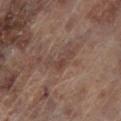This lesion was catalogued during total-body skin photography and was not selected for biopsy. From the left lower leg. A male patient, aged 63–67. A 15 mm crop from a total-body photograph taken for skin-cancer surveillance.